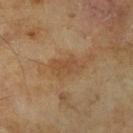biopsy status — imaged on a skin check; not biopsied
TBP lesion metrics — a color-variation rating of about 2.5/10 and peripheral color asymmetry of about 1; an automated nevus-likeness rating near 0 out of 100 and a detector confidence of about 100 out of 100 that the crop contains a lesion
diameter — ≈4 mm
imaging modality — ~15 mm tile from a whole-body skin photo
body site — the right lower leg
patient — male, aged approximately 65
lighting — cross-polarized